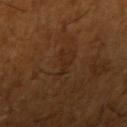  biopsy_status: not biopsied; imaged during a skin examination
  image:
    source: total-body photography crop
    field_of_view_mm: 15
  patient:
    sex: male
    age_approx: 65
  automated_metrics:
    border_irregularity_0_10: 7.0
    color_variation_0_10: 0.0
    nevus_likeness_0_100: 0
    lesion_detection_confidence_0_100: 100
  site: right upper arm
  lesion_size:
    long_diameter_mm_approx: 3.0